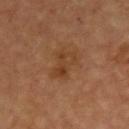Findings:
* follow-up · no biopsy performed (imaged during a skin exam)
* image source · 15 mm crop, total-body photography
* anatomic site · the upper back
* subject · male, aged 63 to 67
* lesion diameter · ~3.5 mm (longest diameter)
* illumination · cross-polarized